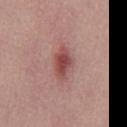workup = imaged on a skin check; not biopsied
imaging modality = ~15 mm tile from a whole-body skin photo
lighting = white-light
patient = male, aged around 30